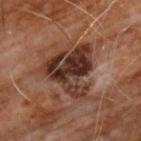Notes:
- follow-up · no biopsy performed (imaged during a skin exam)
- patient · male, aged around 60
- tile lighting · cross-polarized
- image · ~15 mm tile from a whole-body skin photo
- location · the chest
- size · about 7.5 mm